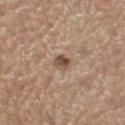Findings:
• notes — imaged on a skin check; not biopsied
• subject — female, aged around 65
• automated metrics — a peripheral color-asymmetry measure near 1; a classifier nevus-likeness of about 50/100 and a lesion-detection confidence of about 100/100
• illumination — white-light illumination
• image — ~15 mm tile from a whole-body skin photo
• diameter — ~2 mm (longest diameter)
• site — the left thigh